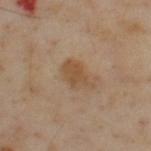No biopsy was performed on this lesion — it was imaged during a full skin examination and was not determined to be concerning.
The lesion is located on the upper back.
Imaged with cross-polarized lighting.
The recorded lesion diameter is about 4 mm.
The patient is a male aged 53 to 57.
Cropped from a total-body skin-imaging series; the visible field is about 15 mm.